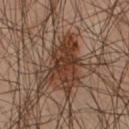Recorded during total-body skin imaging; not selected for excision or biopsy. The lesion's longest dimension is about 6.5 mm. A 15 mm close-up tile from a total-body photography series done for melanoma screening. The lesion-visualizer software estimated an eccentricity of roughly 0.85 and two-axis asymmetry of about 0.45. The analysis additionally found a lesion color around L≈35 a*≈19 b*≈27 in CIELAB, roughly 11 lightness units darker than nearby skin, and a normalized lesion–skin contrast near 10.5. It also reported a border-irregularity rating of about 6/10, a color-variation rating of about 4/10, and peripheral color asymmetry of about 1.5. The analysis additionally found an automated nevus-likeness rating near 90 out of 100 and lesion-presence confidence of about 100/100. A male subject approximately 35 years of age. Captured under cross-polarized illumination. The lesion is located on the chest.